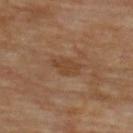No biopsy was performed on this lesion — it was imaged during a full skin examination and was not determined to be concerning. From the upper back. Cropped from a whole-body photographic skin survey; the tile spans about 15 mm. The total-body-photography lesion software estimated a footprint of about 4.5 mm², a shape eccentricity near 0.85, and a symmetry-axis asymmetry near 0.3. It also reported a lesion-detection confidence of about 100/100. A male subject, aged 68–72. Measured at roughly 3.5 mm in maximum diameter.